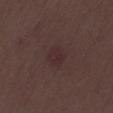Clinical impression:
Imaged during a routine full-body skin examination; the lesion was not biopsied and no histopathology is available.
Context:
Approximately 2.5 mm at its widest. The patient is a male approximately 50 years of age. The tile uses white-light illumination. A lesion tile, about 15 mm wide, cut from a 3D total-body photograph.